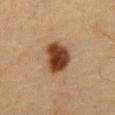Clinical impression:
This lesion was catalogued during total-body skin photography and was not selected for biopsy.
Acquisition and patient details:
The lesion is located on the abdomen. An algorithmic analysis of the crop reported an area of roughly 11 mm², an eccentricity of roughly 0.75, and a symmetry-axis asymmetry near 0.25. And it measured an average lesion color of about L≈32 a*≈18 b*≈27 (CIELAB), about 15 CIELAB-L* units darker than the surrounding skin, and a lesion-to-skin contrast of about 13.5 (normalized; higher = more distinct). The patient is a male aged 58 to 62. A 15 mm close-up extracted from a 3D total-body photography capture. Measured at roughly 4.5 mm in maximum diameter.